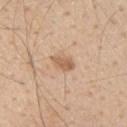Captured during whole-body skin photography for melanoma surveillance; the lesion was not biopsied.
An algorithmic analysis of the crop reported a border-irregularity rating of about 2.5/10 and radial color variation of about 1. It also reported lesion-presence confidence of about 100/100.
A male subject in their mid-50s.
The lesion's longest dimension is about 3 mm.
The lesion is located on the arm.
Captured under white-light illumination.
A region of skin cropped from a whole-body photographic capture, roughly 15 mm wide.